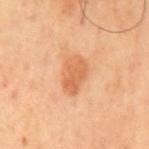Imaged during a routine full-body skin examination; the lesion was not biopsied and no histopathology is available.
From the mid back.
Cropped from a total-body skin-imaging series; the visible field is about 15 mm.
A male subject, aged around 65.